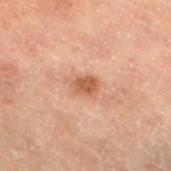Q: Was this lesion biopsied?
A: catalogued during a skin exam; not biopsied
Q: What is the anatomic site?
A: the left lower leg
Q: What are the patient's age and sex?
A: male, approximately 70 years of age
Q: What is the imaging modality?
A: ~15 mm crop, total-body skin-cancer survey